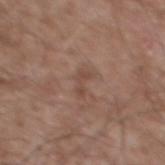<case>
<biopsy_status>not biopsied; imaged during a skin examination</biopsy_status>
<lesion_size>
  <long_diameter_mm_approx>3.0</long_diameter_mm_approx>
</lesion_size>
<site>mid back</site>
<lighting>white-light</lighting>
<patient>
  <sex>male</sex>
  <age_approx>55</age_approx>
</patient>
<automated_metrics>
  <cielab_L>46</cielab_L>
  <cielab_a>19</cielab_a>
  <cielab_b>26</cielab_b>
  <vs_skin_contrast_norm>5.5</vs_skin_contrast_norm>
</automated_metrics>
<image>
  <source>total-body photography crop</source>
  <field_of_view_mm>15</field_of_view_mm>
</image>
</case>